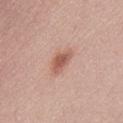Assessment: No biopsy was performed on this lesion — it was imaged during a full skin examination and was not determined to be concerning. Context: Cropped from a whole-body photographic skin survey; the tile spans about 15 mm. The lesion is located on the abdomen. A female subject about 25 years old.